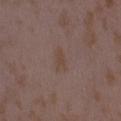biopsy status — catalogued during a skin exam; not biopsied | image-analysis metrics — a footprint of about 4 mm², an outline eccentricity of about 0.8 (0 = round, 1 = elongated), and two-axis asymmetry of about 0.35; a mean CIELAB color near L≈43 a*≈15 b*≈23, roughly 4 lightness units darker than nearby skin, and a normalized lesion–skin contrast near 5.5; a classifier nevus-likeness of about 0/100 and a lesion-detection confidence of about 100/100 | body site — the arm | subject — female, aged 33–37 | lesion size — ≈2.5 mm | lighting — white-light | image source — ~15 mm tile from a whole-body skin photo.